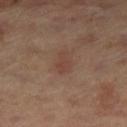The lesion was photographed on a routine skin check and not biopsied; there is no pathology result. Imaged with cross-polarized lighting. From the left leg. A roughly 15 mm field-of-view crop from a total-body skin photograph. Longest diameter approximately 2.5 mm. The lesion-visualizer software estimated an area of roughly 3 mm², a shape eccentricity near 0.8, and a shape-asymmetry score of about 0.35 (0 = symmetric). The software also gave a border-irregularity index near 3.5/10, a color-variation rating of about 1.5/10, and peripheral color asymmetry of about 0.5. The analysis additionally found a nevus-likeness score of about 10/100 and lesion-presence confidence of about 100/100. The subject is a male aged 63 to 67.